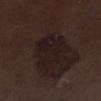notes = total-body-photography surveillance lesion; no biopsy
illumination = white-light
image source = total-body-photography crop, ~15 mm field of view
anatomic site = the left lower leg
TBP lesion metrics = an area of roughly 15 mm², a shape eccentricity near 0.75, and a symmetry-axis asymmetry near 0.3
diameter = about 6 mm
patient = male, approximately 70 years of age